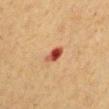Findings:
- follow-up: no biopsy performed (imaged during a skin exam)
- size: about 2.5 mm
- subject: male, about 55 years old
- anatomic site: the left arm
- automated lesion analysis: a lesion area of about 3.5 mm², an eccentricity of roughly 0.8, and a shape-asymmetry score of about 0.25 (0 = symmetric); a mean CIELAB color near L≈45 a*≈30 b*≈32, a lesion–skin lightness drop of about 16, and a lesion-to-skin contrast of about 11.5 (normalized; higher = more distinct); a within-lesion color-variation index near 6.5/10 and a peripheral color-asymmetry measure near 2.5
- imaging modality: total-body-photography crop, ~15 mm field of view